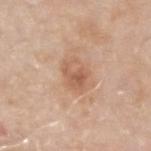Findings:
– workup — catalogued during a skin exam; not biopsied
– patient — male, aged 73 to 77
– lesion diameter — about 3.5 mm
– illumination — white-light illumination
– anatomic site — the left forearm
– image — 15 mm crop, total-body photography